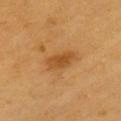biopsy status: catalogued during a skin exam; not biopsied | imaging modality: total-body-photography crop, ~15 mm field of view | image-analysis metrics: a lesion area of about 6 mm² and a shape-asymmetry score of about 0.2 (0 = symmetric); a border-irregularity rating of about 2.5/10 and radial color variation of about 0.5 | patient: male, about 60 years old | lesion size: about 4 mm | site: the chest.